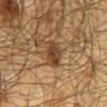The lesion was tiled from a total-body skin photograph and was not biopsied.
A male patient aged approximately 65.
Approximately 3 mm at its widest.
A roughly 15 mm field-of-view crop from a total-body skin photograph.
On the mid back.
Imaged with cross-polarized lighting.